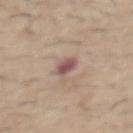Clinical summary:
The patient is a male in their 60s. From the abdomen. Cropped from a total-body skin-imaging series; the visible field is about 15 mm.